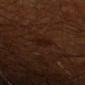workup = imaged on a skin check; not biopsied
size = ≈3 mm
tile lighting = cross-polarized illumination
patient = male, about 70 years old
body site = the right upper arm
image source = total-body-photography crop, ~15 mm field of view